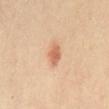The lesion was photographed on a routine skin check and not biopsied; there is no pathology result. About 2.5 mm across. A female patient, roughly 40 years of age. From the abdomen. Cropped from a whole-body photographic skin survey; the tile spans about 15 mm. The total-body-photography lesion software estimated a footprint of about 4 mm², an eccentricity of roughly 0.8, and a symmetry-axis asymmetry near 0.25. It also reported a mean CIELAB color near L≈63 a*≈24 b*≈35 and roughly 11 lightness units darker than nearby skin. Captured under cross-polarized illumination.